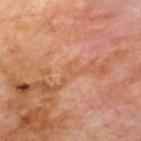Assessment: The lesion was photographed on a routine skin check and not biopsied; there is no pathology result. Context: This is a cross-polarized tile. A 15 mm close-up extracted from a 3D total-body photography capture. On the upper back. The patient is a male aged approximately 70. An algorithmic analysis of the crop reported an automated nevus-likeness rating near 0 out of 100 and a detector confidence of about 100 out of 100 that the crop contains a lesion.